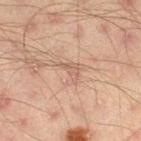Automated image analysis of the tile measured a border-irregularity index near 6.5/10 and a peripheral color-asymmetry measure near 1. This image is a 15 mm lesion crop taken from a total-body photograph. Measured at roughly 3 mm in maximum diameter. The lesion is located on the leg. A male subject, approximately 45 years of age. Captured under cross-polarized illumination.